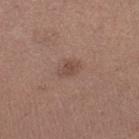Captured during whole-body skin photography for melanoma surveillance; the lesion was not biopsied. The lesion's longest dimension is about 2.5 mm. From the right lower leg. A female patient, roughly 30 years of age. A close-up tile cropped from a whole-body skin photograph, about 15 mm across.